Located on the chest, a roughly 15 mm field-of-view crop from a total-body skin photograph, a female subject aged approximately 60: 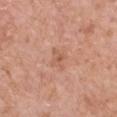Q: Automated lesion metrics?
A: a lesion area of about 4 mm², an eccentricity of roughly 0.65, and a symmetry-axis asymmetry near 0.4; a lesion-to-skin contrast of about 5 (normalized; higher = more distinct); a border-irregularity index near 4/10, a color-variation rating of about 2.5/10, and a peripheral color-asymmetry measure near 1; a classifier nevus-likeness of about 0/100 and a detector confidence of about 100 out of 100 that the crop contains a lesion
Q: How large is the lesion?
A: ≈2.5 mm
Q: How was the tile lit?
A: white-light illumination
Q: What did pathology find?
A: a squamous cell carcinoma in situ (malignant)